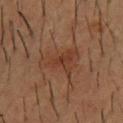Assessment: The lesion was tiled from a total-body skin photograph and was not biopsied. Image and clinical context: A 15 mm close-up tile from a total-body photography series done for melanoma screening. The patient is a male aged 53–57. Automated image analysis of the tile measured an area of roughly 11 mm², an outline eccentricity of about 0.8 (0 = round, 1 = elongated), and a shape-asymmetry score of about 0.45 (0 = symmetric). The analysis additionally found a mean CIELAB color near L≈32 a*≈19 b*≈26, roughly 5 lightness units darker than nearby skin, and a normalized lesion–skin contrast near 5.5. The analysis additionally found a classifier nevus-likeness of about 5/100 and lesion-presence confidence of about 100/100. The recorded lesion diameter is about 5 mm. Imaged with cross-polarized lighting. From the chest.